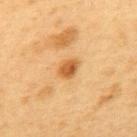Part of a total-body skin-imaging series; this lesion was reviewed on a skin check and was not flagged for biopsy.
Measured at roughly 3 mm in maximum diameter.
This is a cross-polarized tile.
A 15 mm close-up extracted from a 3D total-body photography capture.
A male subject, aged around 55.
The total-body-photography lesion software estimated lesion-presence confidence of about 100/100.
Located on the back.